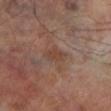automated lesion analysis: an area of roughly 5 mm² and a shape-asymmetry score of about 0.2 (0 = symmetric); border irregularity of about 2 on a 0–10 scale, internal color variation of about 1.5 on a 0–10 scale, and peripheral color asymmetry of about 0.5 | subject: male, aged approximately 70 | lesion diameter: ≈3 mm | acquisition: ~15 mm tile from a whole-body skin photo | body site: the leg.